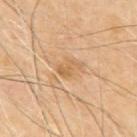Case summary:
– follow-up — catalogued during a skin exam; not biopsied
– location — the mid back
– patient — male, in their 70s
– image — ~15 mm tile from a whole-body skin photo
– lighting — cross-polarized
– size — ~3 mm (longest diameter)